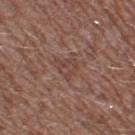notes: imaged on a skin check; not biopsied
anatomic site: the left thigh
diameter: about 2.5 mm
patient: male, aged approximately 45
acquisition: total-body-photography crop, ~15 mm field of view
lighting: white-light illumination